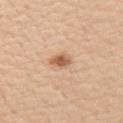This lesion was catalogued during total-body skin photography and was not selected for biopsy. Imaged with white-light lighting. The recorded lesion diameter is about 2.5 mm. Located on the left upper arm. The subject is a male aged 58–62. A region of skin cropped from a whole-body photographic capture, roughly 15 mm wide. Automated tile analysis of the lesion measured a border-irregularity index near 1.5/10 and radial color variation of about 1.